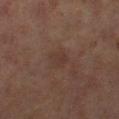biopsy status: imaged on a skin check; not biopsied | size: ≈3 mm | subject: female, aged approximately 60 | illumination: cross-polarized | acquisition: ~15 mm crop, total-body skin-cancer survey | location: the left thigh.